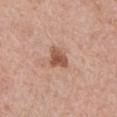Part of a total-body skin-imaging series; this lesion was reviewed on a skin check and was not flagged for biopsy.
Cropped from a total-body skin-imaging series; the visible field is about 15 mm.
From the left upper arm.
A male subject aged approximately 70.